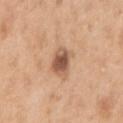Impression: Imaged during a routine full-body skin examination; the lesion was not biopsied and no histopathology is available. Acquisition and patient details: The lesion-visualizer software estimated a mean CIELAB color near L≈55 a*≈21 b*≈31, roughly 14 lightness units darker than nearby skin, and a normalized lesion–skin contrast near 9.5. The software also gave a border-irregularity index near 1.5/10, a within-lesion color-variation index near 4.5/10, and radial color variation of about 1.5. The analysis additionally found a nevus-likeness score of about 50/100 and a detector confidence of about 100 out of 100 that the crop contains a lesion. The recorded lesion diameter is about 2.5 mm. On the right upper arm. Captured under white-light illumination. A female subject aged around 40. A 15 mm close-up tile from a total-body photography series done for melanoma screening.